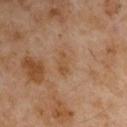Imaged during a routine full-body skin examination; the lesion was not biopsied and no histopathology is available.